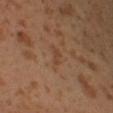notes = catalogued during a skin exam; not biopsied | subject = male, aged approximately 30 | lesion size = about 2.5 mm | illumination = cross-polarized | location = the left leg | imaging modality = ~15 mm crop, total-body skin-cancer survey.